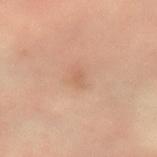{
  "biopsy_status": "not biopsied; imaged during a skin examination",
  "site": "left forearm",
  "lesion_size": {
    "long_diameter_mm_approx": 2.0
  },
  "image": {
    "source": "total-body photography crop",
    "field_of_view_mm": 15
  },
  "automated_metrics": {
    "eccentricity": 0.75,
    "shape_asymmetry": 0.35
  },
  "patient": {
    "sex": "male",
    "age_approx": 50
  }
}